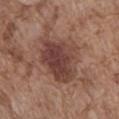imaging modality: ~15 mm tile from a whole-body skin photo; subject: male, aged 73–77; lesion diameter: ≈6 mm; site: the abdomen; tile lighting: white-light.